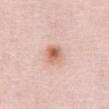<case>
<biopsy_status>not biopsied; imaged during a skin examination</biopsy_status>
<patient>
  <sex>female</sex>
  <age_approx>50</age_approx>
</patient>
<lighting>white-light</lighting>
<automated_metrics>
  <area_mm2_approx>5.5</area_mm2_approx>
  <eccentricity>0.25</eccentricity>
  <border_irregularity_0_10>1.0</border_irregularity_0_10>
  <color_variation_0_10>7.5</color_variation_0_10>
  <nevus_likeness_0_100>90</nevus_likeness_0_100>
  <lesion_detection_confidence_0_100>100</lesion_detection_confidence_0_100>
</automated_metrics>
<site>abdomen</site>
<lesion_size>
  <long_diameter_mm_approx>2.5</long_diameter_mm_approx>
</lesion_size>
<image>
  <source>total-body photography crop</source>
  <field_of_view_mm>15</field_of_view_mm>
</image>
</case>